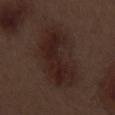The lesion was photographed on a routine skin check and not biopsied; there is no pathology result. Captured under white-light illumination. Cropped from a whole-body photographic skin survey; the tile spans about 15 mm. The lesion is located on the left thigh. A male subject in their 70s. Measured at roughly 8.5 mm in maximum diameter.